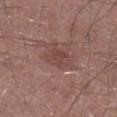Imaged during a routine full-body skin examination; the lesion was not biopsied and no histopathology is available.
A region of skin cropped from a whole-body photographic capture, roughly 15 mm wide.
On the right lower leg.
A male subject aged 68 to 72.
The lesion's longest dimension is about 3.5 mm.
This is a white-light tile.
Automated tile analysis of the lesion measured a lesion area of about 6.5 mm², an eccentricity of roughly 0.75, and two-axis asymmetry of about 0.25.